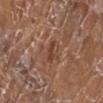On the left lower leg. A 15 mm crop from a total-body photograph taken for skin-cancer surveillance. The recorded lesion diameter is about 2.5 mm. A female patient, in their mid-70s.